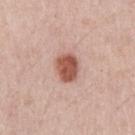Q: Was a biopsy performed?
A: total-body-photography surveillance lesion; no biopsy
Q: How was the tile lit?
A: white-light
Q: What kind of image is this?
A: 15 mm crop, total-body photography
Q: What are the patient's age and sex?
A: male, in their 60s
Q: Where on the body is the lesion?
A: the right upper arm